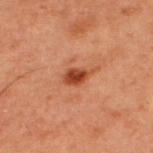Findings:
• notes — imaged on a skin check; not biopsied
• location — the upper back
• patient — male, in their 60s
• imaging modality — ~15 mm crop, total-body skin-cancer survey